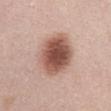follow-up=imaged on a skin check; not biopsied
site=the abdomen
acquisition=~15 mm crop, total-body skin-cancer survey
patient=female, aged 28–32
diameter=~5.5 mm (longest diameter)